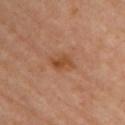Clinical impression:
The lesion was photographed on a routine skin check and not biopsied; there is no pathology result.
Background:
A female patient aged around 60. The lesion is located on the chest. A 15 mm crop from a total-body photograph taken for skin-cancer surveillance. An algorithmic analysis of the crop reported a lesion area of about 5 mm². The software also gave an automated nevus-likeness rating near 15 out of 100 and a detector confidence of about 100 out of 100 that the crop contains a lesion.